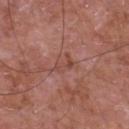Assessment: Recorded during total-body skin imaging; not selected for excision or biopsy. Clinical summary: This image is a 15 mm lesion crop taken from a total-body photograph. The lesion is located on the chest. A male patient in their mid-60s. The tile uses white-light illumination. An algorithmic analysis of the crop reported radial color variation of about 1.5. Longest diameter approximately 3.5 mm.